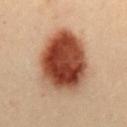The lesion was photographed on a routine skin check and not biopsied; there is no pathology result. An algorithmic analysis of the crop reported an area of roughly 37 mm² and a symmetry-axis asymmetry near 0.15. This is a cross-polarized tile. A 15 mm close-up extracted from a 3D total-body photography capture. A female patient approximately 30 years of age. The lesion is on the mid back.